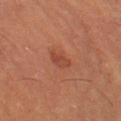biopsy status = imaged on a skin check; not biopsied | subject = male, aged around 55 | diameter = about 2.5 mm | imaging modality = 15 mm crop, total-body photography | tile lighting = cross-polarized illumination | image-analysis metrics = an area of roughly 3.5 mm² and a symmetry-axis asymmetry near 0.35; an average lesion color of about L≈39 a*≈25 b*≈29 (CIELAB), roughly 6 lightness units darker than nearby skin, and a normalized border contrast of about 5.5; a border-irregularity index near 3.5/10, internal color variation of about 2.5 on a 0–10 scale, and a peripheral color-asymmetry measure near 1 | body site = the left lower leg.